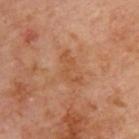Findings:
• notes — total-body-photography surveillance lesion; no biopsy
• subject — male, in their 70s
• lighting — cross-polarized illumination
• image — 15 mm crop, total-body photography
• TBP lesion metrics — a shape eccentricity near 0.9 and a symmetry-axis asymmetry near 0.55; an average lesion color of about L≈51 a*≈23 b*≈36 (CIELAB) and roughly 6 lightness units darker than nearby skin; a border-irregularity index near 7/10, a within-lesion color-variation index near 0.5/10, and a peripheral color-asymmetry measure near 0
• lesion diameter — ~4 mm (longest diameter)
• location — the upper back